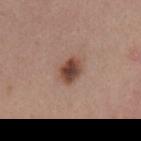| field | value |
|---|---|
| follow-up | no biopsy performed (imaged during a skin exam) |
| acquisition | 15 mm crop, total-body photography |
| lesion diameter | about 3 mm |
| body site | the mid back |
| illumination | white-light illumination |
| subject | female, aged 38 to 42 |